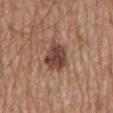| feature | finding |
|---|---|
| notes | no biopsy performed (imaged during a skin exam) |
| lighting | white-light illumination |
| location | the back |
| patient | male, aged 68–72 |
| size | about 5 mm |
| acquisition | 15 mm crop, total-body photography |